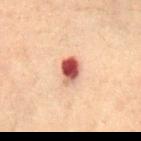biopsy status: catalogued during a skin exam; not biopsied
acquisition: ~15 mm crop, total-body skin-cancer survey
subject: female, about 80 years old
anatomic site: the mid back